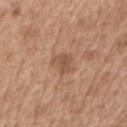Assessment: Captured during whole-body skin photography for melanoma surveillance; the lesion was not biopsied. Context: A male patient, approximately 70 years of age. Approximately 3 mm at its widest. The lesion is located on the right upper arm. This is a white-light tile. A 15 mm close-up extracted from a 3D total-body photography capture.